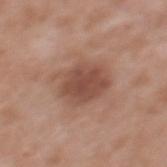follow-up: total-body-photography surveillance lesion; no biopsy
lighting: white-light
patient: female, aged approximately 35
image source: ~15 mm crop, total-body skin-cancer survey
diameter: ≈5.5 mm
automated lesion analysis: a lesion color around L≈49 a*≈21 b*≈27 in CIELAB, roughly 11 lightness units darker than nearby skin, and a lesion-to-skin contrast of about 8 (normalized; higher = more distinct); a border-irregularity rating of about 2.5/10 and a peripheral color-asymmetry measure near 1; a classifier nevus-likeness of about 35/100 and a detector confidence of about 100 out of 100 that the crop contains a lesion
location: the upper back